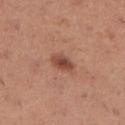This lesion was catalogued during total-body skin photography and was not selected for biopsy.
A roughly 15 mm field-of-view crop from a total-body skin photograph.
The subject is a female about 30 years old.
The lesion is located on the right lower leg.
Approximately 3 mm at its widest.